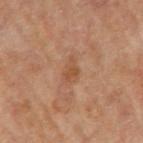Assessment: Recorded during total-body skin imaging; not selected for excision or biopsy. Context: Measured at roughly 3 mm in maximum diameter. The subject is a male aged approximately 70. A 15 mm close-up tile from a total-body photography series done for melanoma screening. From the right upper arm. The tile uses cross-polarized illumination.